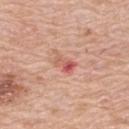A female patient in their mid- to late 60s. A roughly 15 mm field-of-view crop from a total-body skin photograph. Imaged with white-light lighting. The lesion's longest dimension is about 2.5 mm. On the upper back.